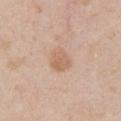Assessment: No biopsy was performed on this lesion — it was imaged during a full skin examination and was not determined to be concerning. Context: The lesion-visualizer software estimated a lesion area of about 6.5 mm², an eccentricity of roughly 0.65, and a symmetry-axis asymmetry near 0.25. It also reported a nevus-likeness score of about 55/100. Cropped from a total-body skin-imaging series; the visible field is about 15 mm. The lesion is located on the chest. The subject is a male roughly 40 years of age. Measured at roughly 3.5 mm in maximum diameter.